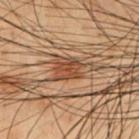{
  "biopsy_status": "not biopsied; imaged during a skin examination",
  "lesion_size": {
    "long_diameter_mm_approx": 3.0
  },
  "site": "chest",
  "automated_metrics": {
    "area_mm2_approx": 5.5,
    "eccentricity": 0.55,
    "shape_asymmetry": 0.3,
    "cielab_L": 37,
    "cielab_a": 19,
    "cielab_b": 28,
    "vs_skin_darker_L": 9.0,
    "vs_skin_contrast_norm": 8.0
  },
  "image": {
    "source": "total-body photography crop",
    "field_of_view_mm": 15
  },
  "patient": {
    "sex": "male",
    "age_approx": 55
  }
}